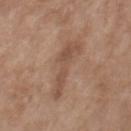  biopsy_status: not biopsied; imaged during a skin examination
  lighting: white-light
  image:
    source: total-body photography crop
    field_of_view_mm: 15
  patient:
    sex: female
    age_approx: 75
  site: chest